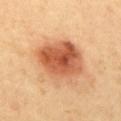Q: Was a biopsy performed?
A: imaged on a skin check; not biopsied
Q: How large is the lesion?
A: ≈6.5 mm
Q: What is the imaging modality?
A: ~15 mm tile from a whole-body skin photo
Q: What is the anatomic site?
A: the mid back
Q: Automated lesion metrics?
A: a footprint of about 24 mm² and a shape-asymmetry score of about 0.15 (0 = symmetric); a lesion–skin lightness drop of about 16; a border-irregularity rating of about 2/10, internal color variation of about 8 on a 0–10 scale, and a peripheral color-asymmetry measure near 2.5
Q: Illumination type?
A: cross-polarized illumination
Q: Who is the patient?
A: female, aged 58 to 62